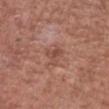Assessment:
The lesion was tiled from a total-body skin photograph and was not biopsied.
Background:
The recorded lesion diameter is about 3 mm. A close-up tile cropped from a whole-body skin photograph, about 15 mm across. A male subject, in their mid-60s. The tile uses white-light illumination. Automated tile analysis of the lesion measured a nevus-likeness score of about 0/100. The lesion is located on the chest.